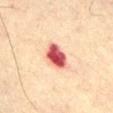Notes:
* biopsy status: no biopsy performed (imaged during a skin exam)
* body site: the front of the torso
* acquisition: ~15 mm crop, total-body skin-cancer survey
* patient: male, in their mid-70s
* tile lighting: cross-polarized
* lesion size: ~3.5 mm (longest diameter)
* image-analysis metrics: an area of roughly 7 mm², an eccentricity of roughly 0.7, and a symmetry-axis asymmetry near 0.2; an average lesion color of about L≈49 a*≈33 b*≈25 (CIELAB) and about 21 CIELAB-L* units darker than the surrounding skin; border irregularity of about 2 on a 0–10 scale, internal color variation of about 5.5 on a 0–10 scale, and peripheral color asymmetry of about 2; lesion-presence confidence of about 100/100